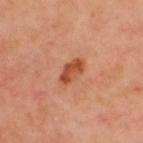  biopsy_status: not biopsied; imaged during a skin examination
  lesion_size:
    long_diameter_mm_approx: 3.5
  site: upper back
  automated_metrics:
    area_mm2_approx: 5.5
    eccentricity: 0.8
    shape_asymmetry: 0.25
    cielab_L: 51
    cielab_a: 29
    cielab_b: 37
    vs_skin_darker_L: 11.0
    vs_skin_contrast_norm: 8.5
    color_variation_0_10: 3.0
    peripheral_color_asymmetry: 1.0
  lighting: cross-polarized
  patient:
    sex: male
    age_approx: 65
  image:
    source: total-body photography crop
    field_of_view_mm: 15